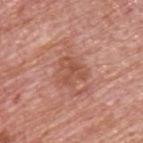Clinical impression:
Recorded during total-body skin imaging; not selected for excision or biopsy.
Image and clinical context:
A male patient approximately 75 years of age. The lesion is on the upper back. Measured at roughly 3.5 mm in maximum diameter. A roughly 15 mm field-of-view crop from a total-body skin photograph. The tile uses white-light illumination.